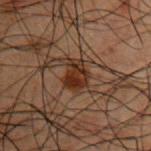Impression: No biopsy was performed on this lesion — it was imaged during a full skin examination and was not determined to be concerning. Acquisition and patient details: The recorded lesion diameter is about 3 mm. A male patient, about 50 years old. The tile uses cross-polarized illumination. The lesion-visualizer software estimated a lesion color around L≈22 a*≈16 b*≈23 in CIELAB, roughly 9 lightness units darker than nearby skin, and a normalized lesion–skin contrast near 10.5. A close-up tile cropped from a whole-body skin photograph, about 15 mm across. The lesion is located on the right upper arm.